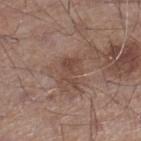| key | value |
|---|---|
| follow-up | imaged on a skin check; not biopsied |
| patient | male, roughly 55 years of age |
| TBP lesion metrics | a nevus-likeness score of about 0/100 and lesion-presence confidence of about 100/100 |
| diameter | ≈3.5 mm |
| tile lighting | white-light |
| image source | ~15 mm tile from a whole-body skin photo |
| location | the right lower leg |